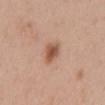Recorded during total-body skin imaging; not selected for excision or biopsy. A female subject, roughly 50 years of age. On the mid back. Cropped from a total-body skin-imaging series; the visible field is about 15 mm.